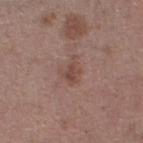Clinical impression:
Captured during whole-body skin photography for melanoma surveillance; the lesion was not biopsied.
Image and clinical context:
A male patient in their mid- to late 60s. Automated tile analysis of the lesion measured an eccentricity of roughly 0.6 and a symmetry-axis asymmetry near 0.35. The software also gave a lesion color around L≈47 a*≈19 b*≈23 in CIELAB, roughly 7 lightness units darker than nearby skin, and a normalized border contrast of about 5.5. It also reported a border-irregularity rating of about 3.5/10 and radial color variation of about 1. The software also gave an automated nevus-likeness rating near 5 out of 100 and a lesion-detection confidence of about 100/100. Measured at roughly 3 mm in maximum diameter. A region of skin cropped from a whole-body photographic capture, roughly 15 mm wide. The tile uses white-light illumination. The lesion is on the left lower leg.